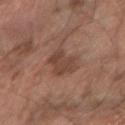Recorded during total-body skin imaging; not selected for excision or biopsy.
About 4.5 mm across.
Captured under cross-polarized illumination.
From the right forearm.
Cropped from a total-body skin-imaging series; the visible field is about 15 mm.
A male patient, aged 63–67.
Automated image analysis of the tile measured an area of roughly 9 mm², a shape eccentricity near 0.8, and two-axis asymmetry of about 0.3. And it measured an average lesion color of about L≈40 a*≈18 b*≈24 (CIELAB), about 8 CIELAB-L* units darker than the surrounding skin, and a normalized lesion–skin contrast near 7. It also reported an automated nevus-likeness rating near 10 out of 100 and a detector confidence of about 100 out of 100 that the crop contains a lesion.